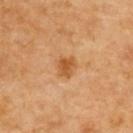The lesion was photographed on a routine skin check and not biopsied; there is no pathology result. On the upper back. Longest diameter approximately 3 mm. A roughly 15 mm field-of-view crop from a total-body skin photograph. A female subject aged 63 to 67.